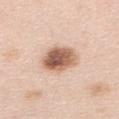Q: What are the patient's age and sex?
A: female, aged 63–67
Q: What is the imaging modality?
A: ~15 mm crop, total-body skin-cancer survey
Q: Lesion size?
A: about 5.5 mm
Q: Automated lesion metrics?
A: a mean CIELAB color near L≈61 a*≈20 b*≈30, roughly 17 lightness units darker than nearby skin, and a lesion-to-skin contrast of about 10.5 (normalized; higher = more distinct)
Q: What lighting was used for the tile?
A: white-light
Q: Lesion location?
A: the upper back